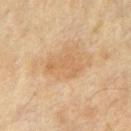No biopsy was performed on this lesion — it was imaged during a full skin examination and was not determined to be concerning. Cropped from a whole-body photographic skin survey; the tile spans about 15 mm. On the chest. The lesion-visualizer software estimated a lesion area of about 6.5 mm², an eccentricity of roughly 0.85, and two-axis asymmetry of about 0.55. It also reported a classifier nevus-likeness of about 0/100. A male subject, in their mid-60s. Approximately 4 mm at its widest.